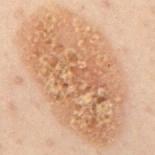biopsy_status: not biopsied; imaged during a skin examination
lesion_size:
  long_diameter_mm_approx: 14.5
image:
  source: total-body photography crop
  field_of_view_mm: 15
site: mid back
patient:
  sex: male
  age_approx: 50